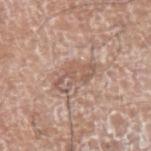Clinical impression: Recorded during total-body skin imaging; not selected for excision or biopsy.